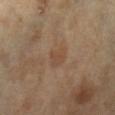follow-up — catalogued during a skin exam; not biopsied | body site — the left leg | imaging modality — ~15 mm crop, total-body skin-cancer survey | illumination — cross-polarized illumination | subject — female, aged around 65 | lesion size — about 2.5 mm.